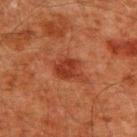Acquisition and patient details: About 3 mm across. A close-up tile cropped from a whole-body skin photograph, about 15 mm across. From the upper back. A male subject roughly 60 years of age. The tile uses cross-polarized illumination.